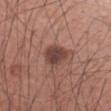No biopsy was performed on this lesion — it was imaged during a full skin examination and was not determined to be concerning. A male subject, aged 53–57. A close-up tile cropped from a whole-body skin photograph, about 15 mm across. Located on the right forearm.